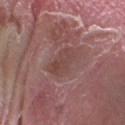No biopsy was performed on this lesion — it was imaged during a full skin examination and was not determined to be concerning.
From the right forearm.
Longest diameter approximately 3.5 mm.
A male subject aged approximately 40.
This is a white-light tile.
A close-up tile cropped from a whole-body skin photograph, about 15 mm across.